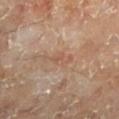Q: Was a biopsy performed?
A: total-body-photography surveillance lesion; no biopsy
Q: Automated lesion metrics?
A: a symmetry-axis asymmetry near 0.45; an automated nevus-likeness rating near 0 out of 100 and lesion-presence confidence of about 95/100
Q: What is the imaging modality?
A: ~15 mm tile from a whole-body skin photo
Q: What is the anatomic site?
A: the left lower leg
Q: Lesion size?
A: about 4 mm
Q: What lighting was used for the tile?
A: cross-polarized illumination
Q: Patient demographics?
A: aged 53 to 57